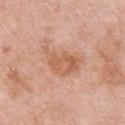biopsy status = total-body-photography surveillance lesion; no biopsy | size = ~5 mm (longest diameter) | patient = male, roughly 55 years of age | tile lighting = white-light | site = the left upper arm | acquisition = 15 mm crop, total-body photography.